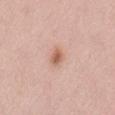A roughly 15 mm field-of-view crop from a total-body skin photograph. The lesion is on the back. Imaged with white-light lighting. Automated image analysis of the tile measured a footprint of about 4 mm² and a shape-asymmetry score of about 0.15 (0 = symmetric). It also reported a mean CIELAB color near L≈62 a*≈22 b*≈30, a lesion–skin lightness drop of about 11, and a lesion-to-skin contrast of about 7.5 (normalized; higher = more distinct). The software also gave border irregularity of about 1.5 on a 0–10 scale and peripheral color asymmetry of about 1. It also reported an automated nevus-likeness rating near 90 out of 100 and a lesion-detection confidence of about 100/100. A female patient in their mid-60s.